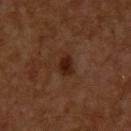Q: What is the imaging modality?
A: ~15 mm tile from a whole-body skin photo
Q: Automated lesion metrics?
A: a lesion area of about 5 mm², an outline eccentricity of about 0.75 (0 = round, 1 = elongated), and two-axis asymmetry of about 0.25; a border-irregularity rating of about 2.5/10, internal color variation of about 3 on a 0–10 scale, and a peripheral color-asymmetry measure near 1
Q: Where on the body is the lesion?
A: the upper back
Q: How was the tile lit?
A: cross-polarized
Q: How large is the lesion?
A: ≈3 mm
Q: What are the patient's age and sex?
A: male, aged around 60